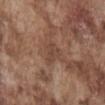The lesion was tiled from a total-body skin photograph and was not biopsied. From the chest. A lesion tile, about 15 mm wide, cut from a 3D total-body photograph. This is a white-light tile. The subject is a male aged 73 to 77. Automated image analysis of the tile measured an eccentricity of roughly 0.75 and two-axis asymmetry of about 0.4. And it measured a border-irregularity index near 3.5/10 and radial color variation of about 0.5. The recorded lesion diameter is about 3 mm.